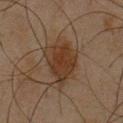Impression: The lesion was tiled from a total-body skin photograph and was not biopsied. Background: This is a cross-polarized tile. A male patient roughly 45 years of age. A 15 mm close-up tile from a total-body photography series done for melanoma screening. From the chest. An algorithmic analysis of the crop reported a mean CIELAB color near L≈28 a*≈14 b*≈24, a lesion–skin lightness drop of about 7, and a normalized border contrast of about 8.5.